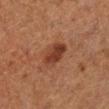biopsy status = imaged on a skin check; not biopsied | anatomic site = the leg | TBP lesion metrics = a mean CIELAB color near L≈31 a*≈21 b*≈26, roughly 9 lightness units darker than nearby skin, and a normalized border contrast of about 8.5 | lighting = cross-polarized | image = ~15 mm crop, total-body skin-cancer survey | lesion size = ~4 mm (longest diameter) | subject = female, about 50 years old.